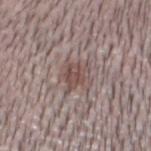Impression:
Captured during whole-body skin photography for melanoma surveillance; the lesion was not biopsied.
Background:
The lesion-visualizer software estimated an average lesion color of about L≈49 a*≈15 b*≈20 (CIELAB), about 9 CIELAB-L* units darker than the surrounding skin, and a normalized border contrast of about 7. And it measured a border-irregularity index near 4.5/10, a color-variation rating of about 4/10, and peripheral color asymmetry of about 1.5. It also reported an automated nevus-likeness rating near 75 out of 100 and lesion-presence confidence of about 65/100. A male subject aged 28 to 32. From the head or neck. Longest diameter approximately 4 mm. A region of skin cropped from a whole-body photographic capture, roughly 15 mm wide. This is a white-light tile.